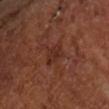This lesion was catalogued during total-body skin photography and was not selected for biopsy. The patient is a male approximately 65 years of age. Measured at roughly 2.5 mm in maximum diameter. Automated tile analysis of the lesion measured border irregularity of about 3 on a 0–10 scale and radial color variation of about 1. The software also gave a detector confidence of about 100 out of 100 that the crop contains a lesion. Located on the arm. This image is a 15 mm lesion crop taken from a total-body photograph.